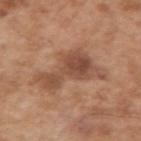biopsy status: imaged on a skin check; not biopsied
imaging modality: ~15 mm crop, total-body skin-cancer survey
lesion size: about 7 mm
illumination: white-light
TBP lesion metrics: an average lesion color of about L≈49 a*≈21 b*≈31 (CIELAB), about 10 CIELAB-L* units darker than the surrounding skin, and a normalized border contrast of about 7.5; a nevus-likeness score of about 25/100 and a lesion-detection confidence of about 100/100
body site: the right upper arm
patient: male, in their mid- to late 50s